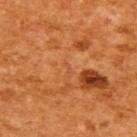This lesion was catalogued during total-body skin photography and was not selected for biopsy.
A female patient aged 53–57.
A region of skin cropped from a whole-body photographic capture, roughly 15 mm wide.
The tile uses cross-polarized illumination.
The lesion's longest dimension is about 1.5 mm.
Automated image analysis of the tile measured a border-irregularity rating of about 4.5/10, a within-lesion color-variation index near 0/10, and a peripheral color-asymmetry measure near 0.
On the back.